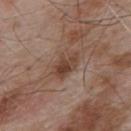Captured during whole-body skin photography for melanoma surveillance; the lesion was not biopsied.
The lesion is on the upper back.
The total-body-photography lesion software estimated an average lesion color of about L≈43 a*≈18 b*≈27 (CIELAB), roughly 10 lightness units darker than nearby skin, and a normalized lesion–skin contrast near 8. And it measured a nevus-likeness score of about 20/100.
A roughly 15 mm field-of-view crop from a total-body skin photograph.
Measured at roughly 4 mm in maximum diameter.
Captured under white-light illumination.
The patient is a male aged around 55.